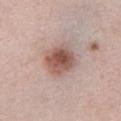biopsy status: imaged on a skin check; not biopsied
tile lighting: white-light illumination
lesion diameter: ~4 mm (longest diameter)
patient: female, roughly 60 years of age
site: the front of the torso
image source: ~15 mm tile from a whole-body skin photo